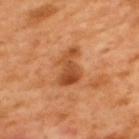Imaged during a routine full-body skin examination; the lesion was not biopsied and no histopathology is available. An algorithmic analysis of the crop reported an area of roughly 8.5 mm² and an eccentricity of roughly 0.85. It also reported about 11 CIELAB-L* units darker than the surrounding skin and a normalized border contrast of about 8. Located on the upper back. Approximately 4.5 mm at its widest. The tile uses cross-polarized illumination. The subject is a male aged around 50. A region of skin cropped from a whole-body photographic capture, roughly 15 mm wide.